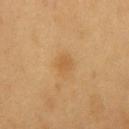Clinical impression: Captured during whole-body skin photography for melanoma surveillance; the lesion was not biopsied. Background: Longest diameter approximately 2.5 mm. Cropped from a whole-body photographic skin survey; the tile spans about 15 mm. A male patient about 60 years old. This is a cross-polarized tile. The lesion is on the chest.